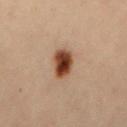Impression: The lesion was tiled from a total-body skin photograph and was not biopsied. Background: A region of skin cropped from a whole-body photographic capture, roughly 15 mm wide. About 3.5 mm across. On the mid back. Captured under cross-polarized illumination. The patient is a female approximately 30 years of age.